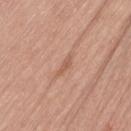Imaged during a routine full-body skin examination; the lesion was not biopsied and no histopathology is available.
From the left thigh.
The subject is a female approximately 40 years of age.
A region of skin cropped from a whole-body photographic capture, roughly 15 mm wide.
The total-body-photography lesion software estimated a shape eccentricity near 0.95 and two-axis asymmetry of about 0.4. The analysis additionally found a border-irregularity rating of about 4/10 and a within-lesion color-variation index near 0/10. The software also gave a nevus-likeness score of about 0/100 and a lesion-detection confidence of about 75/100.
Approximately 3 mm at its widest.
The tile uses white-light illumination.